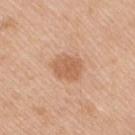Q: Is there a histopathology result?
A: no biopsy performed (imaged during a skin exam)
Q: What did automated image analysis measure?
A: a nevus-likeness score of about 35/100 and a detector confidence of about 100 out of 100 that the crop contains a lesion
Q: What is the anatomic site?
A: the right upper arm
Q: How was this image acquired?
A: 15 mm crop, total-body photography
Q: How was the tile lit?
A: white-light
Q: Lesion size?
A: about 3.5 mm
Q: Patient demographics?
A: male, approximately 70 years of age